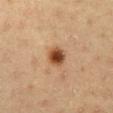Impression:
The lesion was tiled from a total-body skin photograph and was not biopsied.
Background:
Approximately 2.5 mm at its widest. A 15 mm crop from a total-body photograph taken for skin-cancer surveillance. On the abdomen. A male subject aged 58–62.